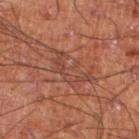The lesion was tiled from a total-body skin photograph and was not biopsied.
The tile uses cross-polarized illumination.
A 15 mm close-up extracted from a 3D total-body photography capture.
The patient is a male aged around 60.
From the left lower leg.
Longest diameter approximately 5.5 mm.